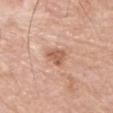diameter: about 2.5 mm | site: the left upper arm | patient: male, about 75 years old | image source: 15 mm crop, total-body photography.